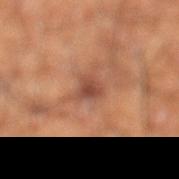Q: Was a biopsy performed?
A: no biopsy performed (imaged during a skin exam)
Q: Lesion location?
A: the left lower leg
Q: What is the imaging modality?
A: 15 mm crop, total-body photography
Q: Who is the patient?
A: male, approximately 60 years of age